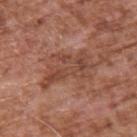The lesion was tiled from a total-body skin photograph and was not biopsied. Measured at roughly 6.5 mm in maximum diameter. Located on the upper back. A lesion tile, about 15 mm wide, cut from a 3D total-body photograph. The lesion-visualizer software estimated an area of roughly 16 mm², an outline eccentricity of about 0.85 (0 = round, 1 = elongated), and two-axis asymmetry of about 0.45. The subject is a male aged approximately 75. Imaged with white-light lighting.